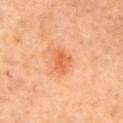The lesion was tiled from a total-body skin photograph and was not biopsied.